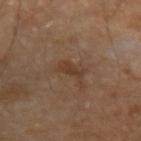Part of a total-body skin-imaging series; this lesion was reviewed on a skin check and was not flagged for biopsy.
On the left forearm.
A close-up tile cropped from a whole-body skin photograph, about 15 mm across.
This is a cross-polarized tile.
A male patient aged around 60.
About 2.5 mm across.
Automated image analysis of the tile measured a lesion area of about 3 mm², an outline eccentricity of about 0.9 (0 = round, 1 = elongated), and a shape-asymmetry score of about 0.35 (0 = symmetric). The software also gave a mean CIELAB color near L≈35 a*≈17 b*≈28, about 7 CIELAB-L* units darker than the surrounding skin, and a lesion-to-skin contrast of about 7 (normalized; higher = more distinct). The analysis additionally found a classifier nevus-likeness of about 0/100 and a lesion-detection confidence of about 100/100.